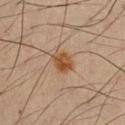Q: Is there a histopathology result?
A: total-body-photography surveillance lesion; no biopsy
Q: What is the anatomic site?
A: the front of the torso
Q: What is the lesion's diameter?
A: ≈3 mm
Q: What kind of image is this?
A: total-body-photography crop, ~15 mm field of view
Q: What are the patient's age and sex?
A: male, aged around 60
Q: What lighting was used for the tile?
A: cross-polarized illumination
Q: Automated lesion metrics?
A: a lesion area of about 5.5 mm² and a shape-asymmetry score of about 0.25 (0 = symmetric); roughly 9 lightness units darker than nearby skin and a lesion-to-skin contrast of about 9.5 (normalized; higher = more distinct); an automated nevus-likeness rating near 95 out of 100 and a detector confidence of about 100 out of 100 that the crop contains a lesion